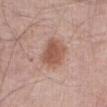Clinical impression:
The lesion was tiled from a total-body skin photograph and was not biopsied.
Context:
The lesion is on the abdomen. The total-body-photography lesion software estimated a shape eccentricity near 0.5 and a shape-asymmetry score of about 0.2 (0 = symmetric). And it measured border irregularity of about 2 on a 0–10 scale, internal color variation of about 3 on a 0–10 scale, and a peripheral color-asymmetry measure near 1. Longest diameter approximately 4 mm. A 15 mm close-up extracted from a 3D total-body photography capture. Imaged with white-light lighting. The subject is a male aged 78 to 82.